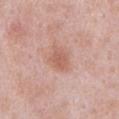{"biopsy_status": "not biopsied; imaged during a skin examination", "lesion_size": {"long_diameter_mm_approx": 3.0}, "image": {"source": "total-body photography crop", "field_of_view_mm": 15}, "automated_metrics": {"cielab_L": 59, "cielab_a": 23, "cielab_b": 28, "vs_skin_contrast_norm": 6.0, "nevus_likeness_0_100": 80, "lesion_detection_confidence_0_100": 100}, "patient": {"sex": "male", "age_approx": 55}, "site": "abdomen"}